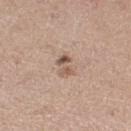follow-up = no biopsy performed (imaged during a skin exam) | image = 15 mm crop, total-body photography | size = ≈3 mm | location = the leg | subject = female, aged around 45 | tile lighting = white-light illumination.